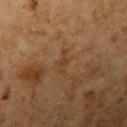Located on the left upper arm. Measured at roughly 3 mm in maximum diameter. A lesion tile, about 15 mm wide, cut from a 3D total-body photograph. The total-body-photography lesion software estimated a border-irregularity index near 4.5/10 and a peripheral color-asymmetry measure near 0. Imaged with cross-polarized lighting. A male subject, aged 58–62.